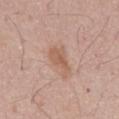workup: no biopsy performed (imaged during a skin exam) | subject: male, about 55 years old | image: ~15 mm tile from a whole-body skin photo | body site: the abdomen.